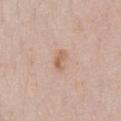{"patient": {"sex": "female", "age_approx": 50}, "site": "front of the torso", "image": {"source": "total-body photography crop", "field_of_view_mm": 15}}